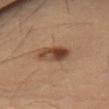Impression:
The lesion was photographed on a routine skin check and not biopsied; there is no pathology result.
Context:
A female subject in their 70s. The lesion is located on the abdomen. About 4 mm across. Imaged with cross-polarized lighting. A 15 mm close-up tile from a total-body photography series done for melanoma screening.